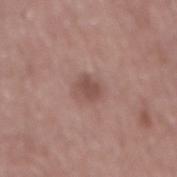Clinical impression:
Part of a total-body skin-imaging series; this lesion was reviewed on a skin check and was not flagged for biopsy.
Acquisition and patient details:
A region of skin cropped from a whole-body photographic capture, roughly 15 mm wide. A male subject aged approximately 60. This is a white-light tile. On the mid back.